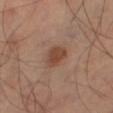Clinical summary:
Longest diameter approximately 3.5 mm. The lesion is on the right thigh. This image is a 15 mm lesion crop taken from a total-body photograph.